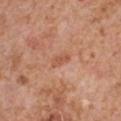workup — no biopsy performed (imaged during a skin exam) | lesion size — ~2.5 mm (longest diameter) | image — ~15 mm crop, total-body skin-cancer survey | patient — male, aged approximately 65 | anatomic site — the chest.